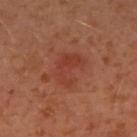biopsy status=no biopsy performed (imaged during a skin exam); lesion size=about 4 mm; patient=female, roughly 40 years of age; location=the right upper arm; image=~15 mm crop, total-body skin-cancer survey.